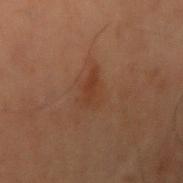biopsy_status: not biopsied; imaged during a skin examination
site: arm
patient:
  sex: male
  age_approx: 55
lighting: cross-polarized
lesion_size:
  long_diameter_mm_approx: 3.5
image:
  source: total-body photography crop
  field_of_view_mm: 15
automated_metrics:
  area_mm2_approx: 5.5
  eccentricity: 0.75
  shape_asymmetry: 0.45
  cielab_L: 30
  cielab_a: 18
  cielab_b: 26
  vs_skin_darker_L: 5.0
  border_irregularity_0_10: 5.0
  peripheral_color_asymmetry: 0.5